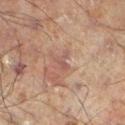Assessment:
No biopsy was performed on this lesion — it was imaged during a full skin examination and was not determined to be concerning.
Background:
The lesion is located on the leg. The subject is a male in their 60s. Cropped from a whole-body photographic skin survey; the tile spans about 15 mm.